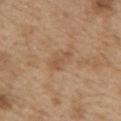| key | value |
|---|---|
| workup | imaged on a skin check; not biopsied |
| body site | the left upper arm |
| acquisition | total-body-photography crop, ~15 mm field of view |
| patient | male, approximately 70 years of age |
| illumination | white-light |